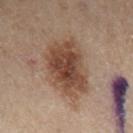A female patient, in their mid- to late 50s.
On the left leg.
Approximately 6.5 mm at its widest.
This is a cross-polarized tile.
A close-up tile cropped from a whole-body skin photograph, about 15 mm across.